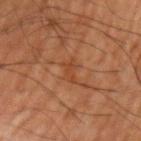Captured during whole-body skin photography for melanoma surveillance; the lesion was not biopsied.